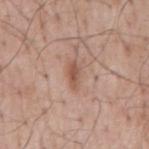The lesion was photographed on a routine skin check and not biopsied; there is no pathology result.
This is a white-light tile.
The lesion is located on the mid back.
A 15 mm crop from a total-body photograph taken for skin-cancer surveillance.
The lesion's longest dimension is about 3 mm.
A male patient aged approximately 60.